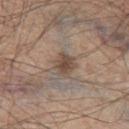The lesion was tiled from a total-body skin photograph and was not biopsied.
A 15 mm crop from a total-body photograph taken for skin-cancer surveillance.
This is a white-light tile.
The patient is a male about 45 years old.
The lesion-visualizer software estimated a lesion area of about 6.5 mm², an eccentricity of roughly 0.45, and a shape-asymmetry score of about 0.25 (0 = symmetric). It also reported lesion-presence confidence of about 100/100.
From the leg.